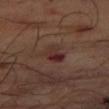The lesion was photographed on a routine skin check and not biopsied; there is no pathology result. The lesion is on the right thigh. A region of skin cropped from a whole-body photographic capture, roughly 15 mm wide. The patient is a male approximately 65 years of age. The lesion's longest dimension is about 3 mm. Imaged with cross-polarized lighting.